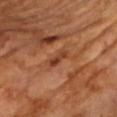Assessment:
No biopsy was performed on this lesion — it was imaged during a full skin examination and was not determined to be concerning.
Acquisition and patient details:
The patient is a male aged approximately 60. On the upper back. Measured at roughly 3 mm in maximum diameter. Automated tile analysis of the lesion measured a footprint of about 2.5 mm², a shape eccentricity near 0.9, and two-axis asymmetry of about 0.3. It also reported an average lesion color of about L≈38 a*≈25 b*≈34 (CIELAB), a lesion–skin lightness drop of about 9, and a normalized border contrast of about 8. And it measured a nevus-likeness score of about 0/100 and a lesion-detection confidence of about 100/100. Cropped from a total-body skin-imaging series; the visible field is about 15 mm. The tile uses cross-polarized illumination.